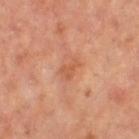Assessment: The lesion was photographed on a routine skin check and not biopsied; there is no pathology result. Context: Automated tile analysis of the lesion measured a border-irregularity index near 4/10 and internal color variation of about 1.5 on a 0–10 scale. It also reported a nevus-likeness score of about 0/100 and a lesion-detection confidence of about 100/100. Located on the left thigh. This is a cross-polarized tile. The subject is a female about 65 years old. Measured at roughly 2.5 mm in maximum diameter. A 15 mm close-up tile from a total-body photography series done for melanoma screening.